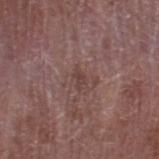Imaged during a routine full-body skin examination; the lesion was not biopsied and no histopathology is available.
A male patient aged 73 to 77.
The lesion's longest dimension is about 2 mm.
A region of skin cropped from a whole-body photographic capture, roughly 15 mm wide.
Located on the right lower leg.